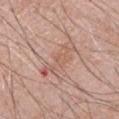This lesion was catalogued during total-body skin photography and was not selected for biopsy. This is a white-light tile. Automated image analysis of the tile measured an eccentricity of roughly 0.95 and a symmetry-axis asymmetry near 0.4. The software also gave a mean CIELAB color near L≈59 a*≈21 b*≈27 and a lesion–skin lightness drop of about 7. The software also gave a color-variation rating of about 3.5/10 and radial color variation of about 0.5. The analysis additionally found a classifier nevus-likeness of about 0/100 and lesion-presence confidence of about 90/100. About 6 mm across. The lesion is located on the chest. A lesion tile, about 15 mm wide, cut from a 3D total-body photograph. A male subject aged around 70.